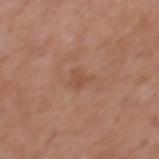Q: Lesion size?
A: about 2.5 mm
Q: Illumination type?
A: white-light illumination
Q: What are the patient's age and sex?
A: male, aged around 70
Q: What is the imaging modality?
A: 15 mm crop, total-body photography
Q: Where on the body is the lesion?
A: the mid back